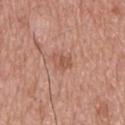This lesion was catalogued during total-body skin photography and was not selected for biopsy. Imaged with white-light lighting. A male patient, roughly 65 years of age. The recorded lesion diameter is about 2.5 mm. A region of skin cropped from a whole-body photographic capture, roughly 15 mm wide. The lesion is on the upper back.